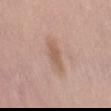No biopsy was performed on this lesion — it was imaged during a full skin examination and was not determined to be concerning. Approximately 4.5 mm at its widest. From the back. Automated tile analysis of the lesion measured an area of roughly 5.5 mm², an outline eccentricity of about 0.95 (0 = round, 1 = elongated), and a shape-asymmetry score of about 0.25 (0 = symmetric). The analysis additionally found a border-irregularity rating of about 3/10 and a within-lesion color-variation index near 1/10. The software also gave a nevus-likeness score of about 0/100. A 15 mm crop from a total-body photograph taken for skin-cancer surveillance. The tile uses white-light illumination. The subject is a female approximately 60 years of age.